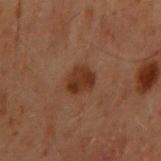Impression:
Captured during whole-body skin photography for melanoma surveillance; the lesion was not biopsied.
Background:
The subject is a male roughly 60 years of age. A 15 mm close-up tile from a total-body photography series done for melanoma screening. The lesion is located on the right upper arm. Automated image analysis of the tile measured a footprint of about 6.5 mm² and a shape eccentricity near 0.55. The software also gave an average lesion color of about L≈25 a*≈17 b*≈24 (CIELAB), about 8 CIELAB-L* units darker than the surrounding skin, and a normalized border contrast of about 9. The analysis additionally found a border-irregularity rating of about 2/10, internal color variation of about 2.5 on a 0–10 scale, and peripheral color asymmetry of about 1. The software also gave a nevus-likeness score of about 55/100. The recorded lesion diameter is about 3 mm.